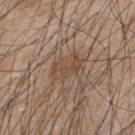Acquisition and patient details: A 15 mm close-up tile from a total-body photography series done for melanoma screening. Imaged with white-light lighting. Longest diameter approximately 3.5 mm. A male subject in their mid-40s. From the upper back.